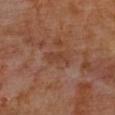workup: imaged on a skin check; not biopsied | acquisition: total-body-photography crop, ~15 mm field of view | lesion size: ≈3 mm | lighting: cross-polarized | automated metrics: roughly 6 lightness units darker than nearby skin and a normalized border contrast of about 6 | site: the back | patient: female.